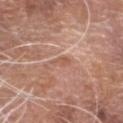Impression: The lesion was photographed on a routine skin check and not biopsied; there is no pathology result. Background: A male subject, aged 73–77. Imaged with white-light lighting. Cropped from a total-body skin-imaging series; the visible field is about 15 mm. Located on the head or neck.